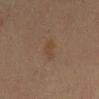workup: catalogued during a skin exam; not biopsied | automated lesion analysis: a footprint of about 3 mm², an outline eccentricity of about 0.95 (0 = round, 1 = elongated), and a symmetry-axis asymmetry near 0.4 | site: the mid back | patient: male, aged approximately 70 | image source: total-body-photography crop, ~15 mm field of view | diameter: ~3.5 mm (longest diameter).